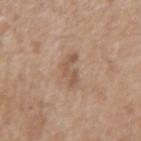The lesion was tiled from a total-body skin photograph and was not biopsied.